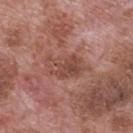notes: no biopsy performed (imaged during a skin exam)
image: 15 mm crop, total-body photography
subject: male, aged around 70
site: the mid back